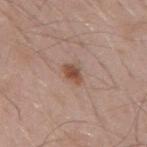Impression: Captured during whole-body skin photography for melanoma surveillance; the lesion was not biopsied. Context: A male subject, aged 53 to 57. From the mid back. A lesion tile, about 15 mm wide, cut from a 3D total-body photograph.